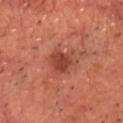workup — imaged on a skin check; not biopsied | automated metrics — a lesion area of about 5.5 mm², an outline eccentricity of about 0.4 (0 = round, 1 = elongated), and a shape-asymmetry score of about 0.25 (0 = symmetric); an average lesion color of about L≈41 a*≈28 b*≈31 (CIELAB), about 10 CIELAB-L* units darker than the surrounding skin, and a normalized lesion–skin contrast near 8; border irregularity of about 2 on a 0–10 scale, a color-variation rating of about 2/10, and peripheral color asymmetry of about 0.5 | patient — male, aged approximately 70 | lighting — cross-polarized illumination | body site — the chest | diameter — about 2.5 mm | acquisition — ~15 mm crop, total-body skin-cancer survey.